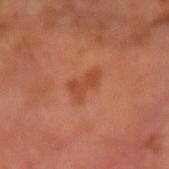Case summary:
– workup · imaged on a skin check; not biopsied
– acquisition · 15 mm crop, total-body photography
– TBP lesion metrics · an area of roughly 5.5 mm², a shape eccentricity near 0.85, and a symmetry-axis asymmetry near 0.4; a border-irregularity rating of about 4.5/10, a color-variation rating of about 2.5/10, and peripheral color asymmetry of about 1
– lighting · cross-polarized illumination
– patient · male, approximately 65 years of age
– lesion diameter · ~3.5 mm (longest diameter)